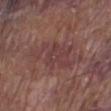{
  "biopsy_status": "not biopsied; imaged during a skin examination",
  "patient": {
    "sex": "male",
    "age_approx": 80
  },
  "automated_metrics": {
    "area_mm2_approx": 14.0,
    "shape_asymmetry": 0.45,
    "cielab_L": 40,
    "cielab_a": 21,
    "cielab_b": 19,
    "vs_skin_darker_L": 6.0,
    "vs_skin_contrast_norm": 6.0,
    "color_variation_0_10": 2.5,
    "peripheral_color_asymmetry": 1.0
  },
  "site": "left lower leg",
  "lesion_size": {
    "long_diameter_mm_approx": 6.5
  },
  "image": {
    "source": "total-body photography crop",
    "field_of_view_mm": 15
  },
  "lighting": "white-light"
}